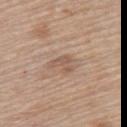| field | value |
|---|---|
| workup | catalogued during a skin exam; not biopsied |
| subject | female, in their mid- to late 60s |
| illumination | white-light |
| anatomic site | the upper back |
| lesion size | ~3 mm (longest diameter) |
| automated lesion analysis | a mean CIELAB color near L≈56 a*≈18 b*≈28, roughly 8 lightness units darker than nearby skin, and a lesion-to-skin contrast of about 5.5 (normalized; higher = more distinct) |
| acquisition | 15 mm crop, total-body photography |